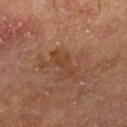This lesion was catalogued during total-body skin photography and was not selected for biopsy. The lesion is on the leg. A 15 mm crop from a total-body photograph taken for skin-cancer surveillance. A male subject, roughly 65 years of age. Automated image analysis of the tile measured a footprint of about 5 mm², a shape eccentricity near 0.9, and a symmetry-axis asymmetry near 0.4. The software also gave an average lesion color of about L≈39 a*≈21 b*≈29 (CIELAB) and roughly 6 lightness units darker than nearby skin.